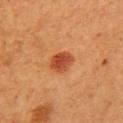The lesion was tiled from a total-body skin photograph and was not biopsied.
Captured under cross-polarized illumination.
This image is a 15 mm lesion crop taken from a total-body photograph.
On the chest.
A female subject, roughly 50 years of age.
Measured at roughly 3 mm in maximum diameter.